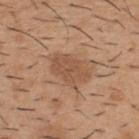lesion diameter=about 4.5 mm
acquisition=total-body-photography crop, ~15 mm field of view
subject=male, aged 38–42
body site=the back
lighting=white-light